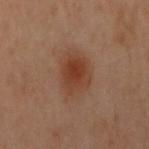notes = imaged on a skin check; not biopsied | patient = female, aged approximately 60 | image source = ~15 mm crop, total-body skin-cancer survey | body site = the left arm.